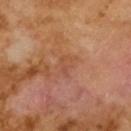| key | value |
|---|---|
| size | ≈3 mm |
| tile lighting | cross-polarized illumination |
| image | 15 mm crop, total-body photography |
| TBP lesion metrics | a lesion color around L≈48 a*≈23 b*≈32 in CIELAB, roughly 5 lightness units darker than nearby skin, and a lesion-to-skin contrast of about 4 (normalized; higher = more distinct); a border-irregularity index near 4/10, internal color variation of about 1.5 on a 0–10 scale, and a peripheral color-asymmetry measure near 0; lesion-presence confidence of about 100/100 |
| subject | male, in their mid- to late 60s |